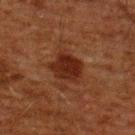{
  "biopsy_status": "not biopsied; imaged during a skin examination",
  "site": "upper back",
  "lighting": "cross-polarized",
  "image": {
    "source": "total-body photography crop",
    "field_of_view_mm": 15
  },
  "lesion_size": {
    "long_diameter_mm_approx": 4.0
  },
  "patient": {
    "sex": "male",
    "age_approx": 60
  }
}